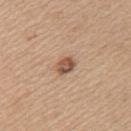The lesion was photographed on a routine skin check and not biopsied; there is no pathology result.
The lesion is located on the left upper arm.
This is a white-light tile.
A female patient aged around 45.
A roughly 15 mm field-of-view crop from a total-body skin photograph.